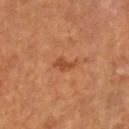Impression: Part of a total-body skin-imaging series; this lesion was reviewed on a skin check and was not flagged for biopsy. Background: An algorithmic analysis of the crop reported an average lesion color of about L≈47 a*≈27 b*≈37 (CIELAB), a lesion–skin lightness drop of about 8, and a lesion-to-skin contrast of about 6.5 (normalized; higher = more distinct). It also reported internal color variation of about 1 on a 0–10 scale and peripheral color asymmetry of about 0.5. A close-up tile cropped from a whole-body skin photograph, about 15 mm across. Longest diameter approximately 3 mm. Captured under cross-polarized illumination. From the left leg. A male subject, approximately 65 years of age.